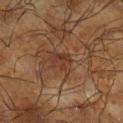<record>
<biopsy_status>not biopsied; imaged during a skin examination</biopsy_status>
<site>right lower leg</site>
<patient>
  <sex>male</sex>
  <age_approx>65</age_approx>
</patient>
<image>
  <source>total-body photography crop</source>
  <field_of_view_mm>15</field_of_view_mm>
</image>
</record>